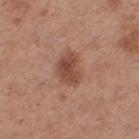| feature | finding |
|---|---|
| patient | female, aged approximately 30 |
| location | the leg |
| image | ~15 mm tile from a whole-body skin photo |